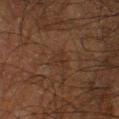Q: Was this lesion biopsied?
A: catalogued during a skin exam; not biopsied
Q: What is the anatomic site?
A: the left thigh
Q: Automated lesion metrics?
A: a lesion area of about 3 mm², a shape eccentricity near 0.85, and a symmetry-axis asymmetry near 0.35; a lesion–skin lightness drop of about 4 and a normalized lesion–skin contrast near 4.5; border irregularity of about 4 on a 0–10 scale, a color-variation rating of about 1.5/10, and a peripheral color-asymmetry measure near 0.5; a nevus-likeness score of about 0/100 and lesion-presence confidence of about 90/100
Q: Lesion size?
A: about 2.5 mm
Q: What kind of image is this?
A: 15 mm crop, total-body photography
Q: Patient demographics?
A: male, in their mid-60s